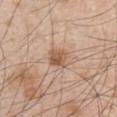Part of a total-body skin-imaging series; this lesion was reviewed on a skin check and was not flagged for biopsy. A male patient aged 48 to 52. An algorithmic analysis of the crop reported a lesion area of about 5.5 mm², an outline eccentricity of about 0.4 (0 = round, 1 = elongated), and two-axis asymmetry of about 0.2. The software also gave a mean CIELAB color near L≈57 a*≈19 b*≈31, roughly 11 lightness units darker than nearby skin, and a normalized lesion–skin contrast near 7.5. The software also gave border irregularity of about 2 on a 0–10 scale, internal color variation of about 4 on a 0–10 scale, and radial color variation of about 1.5. Cropped from a total-body skin-imaging series; the visible field is about 15 mm. From the arm. Approximately 2.5 mm at its widest. The tile uses white-light illumination.